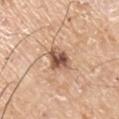Image and clinical context: The lesion's longest dimension is about 3.5 mm. The lesion is on the right upper arm. The subject is a male in their mid- to late 70s. A roughly 15 mm field-of-view crop from a total-body skin photograph.